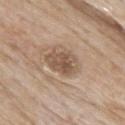Assessment:
Imaged during a routine full-body skin examination; the lesion was not biopsied and no histopathology is available.
Background:
Cropped from a total-body skin-imaging series; the visible field is about 15 mm. The lesion is on the upper back. A male subject in their mid- to late 80s or older. About 4.5 mm across.